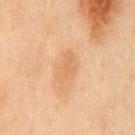<case>
<lesion_size>
  <long_diameter_mm_approx>4.0</long_diameter_mm_approx>
</lesion_size>
<site>front of the torso</site>
<image>
  <source>total-body photography crop</source>
  <field_of_view_mm>15</field_of_view_mm>
</image>
<patient>
  <sex>male</sex>
  <age_approx>45</age_approx>
</patient>
</case>